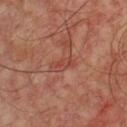biopsy_status: not biopsied; imaged during a skin examination